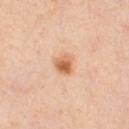Part of a total-body skin-imaging series; this lesion was reviewed on a skin check and was not flagged for biopsy.
The lesion is on the leg.
The subject is a female approximately 40 years of age.
Measured at roughly 2.5 mm in maximum diameter.
A close-up tile cropped from a whole-body skin photograph, about 15 mm across.